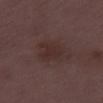<tbp_lesion>
<patient>
  <sex>female</sex>
  <age_approx>30</age_approx>
</patient>
<lighting>white-light</lighting>
<automated_metrics>
  <area_mm2_approx>7.5</area_mm2_approx>
  <cielab_L>28</cielab_L>
  <cielab_a>17</cielab_a>
  <cielab_b>17</cielab_b>
  <vs_skin_darker_L>5.0</vs_skin_darker_L>
  <vs_skin_contrast_norm>6.0</vs_skin_contrast_norm>
</automated_metrics>
<site>right thigh</site>
<image>
  <source>total-body photography crop</source>
  <field_of_view_mm>15</field_of_view_mm>
</image>
<lesion_size>
  <long_diameter_mm_approx>3.5</long_diameter_mm_approx>
</lesion_size>
</tbp_lesion>